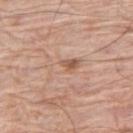Background:
The lesion-visualizer software estimated a footprint of about 6.5 mm², a shape eccentricity near 0.9, and two-axis asymmetry of about 0.3. The analysis additionally found a normalized lesion–skin contrast near 4.5. The analysis additionally found a classifier nevus-likeness of about 0/100. On the right thigh. This is a white-light tile. The patient is a male about 80 years old. A region of skin cropped from a whole-body photographic capture, roughly 15 mm wide. The lesion's longest dimension is about 4.5 mm.